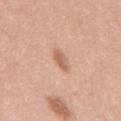Clinical impression: Recorded during total-body skin imaging; not selected for excision or biopsy. Acquisition and patient details: On the mid back. Cropped from a whole-body photographic skin survey; the tile spans about 15 mm. The lesion's longest dimension is about 2.5 mm. Imaged with white-light lighting. A male subject aged 43 to 47.